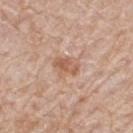body site: the upper back | imaging modality: ~15 mm tile from a whole-body skin photo | patient: male, in their 80s | TBP lesion metrics: an area of roughly 4.5 mm² and a shape-asymmetry score of about 0.3 (0 = symmetric); an automated nevus-likeness rating near 10 out of 100 and a detector confidence of about 100 out of 100 that the crop contains a lesion.